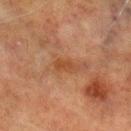Part of a total-body skin-imaging series; this lesion was reviewed on a skin check and was not flagged for biopsy. Captured under cross-polarized illumination. Longest diameter approximately 3 mm. A male subject, in their 70s. A 15 mm close-up extracted from a 3D total-body photography capture. The lesion is on the left lower leg. An algorithmic analysis of the crop reported a lesion area of about 3.5 mm², an eccentricity of roughly 0.9, and a shape-asymmetry score of about 0.35 (0 = symmetric). The software also gave a lesion color around L≈39 a*≈21 b*≈32 in CIELAB, roughly 6 lightness units darker than nearby skin, and a normalized border contrast of about 6.5. And it measured a border-irregularity index near 3.5/10 and peripheral color asymmetry of about 0.5. The analysis additionally found lesion-presence confidence of about 100/100.